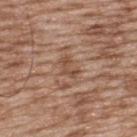Part of a total-body skin-imaging series; this lesion was reviewed on a skin check and was not flagged for biopsy.
Imaged with white-light lighting.
The patient is a male aged around 50.
The lesion-visualizer software estimated border irregularity of about 6 on a 0–10 scale and radial color variation of about 0. It also reported an automated nevus-likeness rating near 0 out of 100.
A lesion tile, about 15 mm wide, cut from a 3D total-body photograph.
The lesion is located on the upper back.